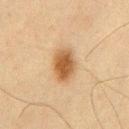biopsy_status: not biopsied; imaged during a skin examination
patient:
  sex: male
  age_approx: 45
image:
  source: total-body photography crop
  field_of_view_mm: 15
site: front of the torso
lighting: cross-polarized